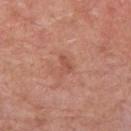Clinical impression:
Recorded during total-body skin imaging; not selected for excision or biopsy.
Clinical summary:
The lesion is on the leg. Cropped from a total-body skin-imaging series; the visible field is about 15 mm. A male subject approximately 80 years of age. An algorithmic analysis of the crop reported an area of roughly 3.5 mm², a shape eccentricity near 0.75, and two-axis asymmetry of about 0.5. The software also gave a nevus-likeness score of about 0/100 and lesion-presence confidence of about 100/100.